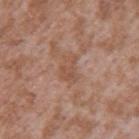Part of a total-body skin-imaging series; this lesion was reviewed on a skin check and was not flagged for biopsy.